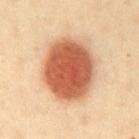Captured during whole-body skin photography for melanoma surveillance; the lesion was not biopsied. A male subject, in their 40s. Imaged with cross-polarized lighting. Located on the abdomen. A 15 mm close-up tile from a total-body photography series done for melanoma screening. About 7 mm across.